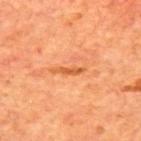| key | value |
|---|---|
| lighting | cross-polarized illumination |
| automated metrics | a lesion color around L≈58 a*≈32 b*≈46 in CIELAB, about 10 CIELAB-L* units darker than the surrounding skin, and a lesion-to-skin contrast of about 7.5 (normalized; higher = more distinct); a border-irregularity index near 3.5/10, a color-variation rating of about 0/10, and a peripheral color-asymmetry measure near 0 |
| lesion size | ~2.5 mm (longest diameter) |
| body site | the upper back |
| patient | male, about 70 years old |
| imaging modality | ~15 mm crop, total-body skin-cancer survey |